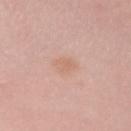automated lesion analysis: a footprint of about 4.5 mm², a shape eccentricity near 0.55, and two-axis asymmetry of about 0.2; an average lesion color of about L≈65 a*≈21 b*≈29 (CIELAB), about 5 CIELAB-L* units darker than the surrounding skin, and a normalized border contrast of about 4.5 | acquisition: total-body-photography crop, ~15 mm field of view | lesion diameter: ~2.5 mm (longest diameter) | tile lighting: white-light illumination | subject: female, aged around 55 | location: the left forearm.